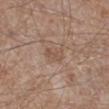The lesion was tiled from a total-body skin photograph and was not biopsied. The tile uses white-light illumination. A male patient approximately 65 years of age. A close-up tile cropped from a whole-body skin photograph, about 15 mm across. The lesion's longest dimension is about 2.5 mm. The lesion is on the right lower leg.